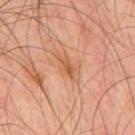Impression: This lesion was catalogued during total-body skin photography and was not selected for biopsy. Background: Imaged with cross-polarized lighting. The lesion is located on the mid back. The lesion's longest dimension is about 4 mm. A close-up tile cropped from a whole-body skin photograph, about 15 mm across. A male subject aged around 45.